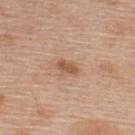Clinical impression: Recorded during total-body skin imaging; not selected for excision or biopsy. Acquisition and patient details: A female patient aged around 65. Cropped from a total-body skin-imaging series; the visible field is about 15 mm. Imaged with white-light lighting. On the upper back.